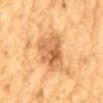workup=catalogued during a skin exam; not biopsied | patient=male, aged approximately 85 or older | location=the back | lesion diameter=≈6.5 mm | lighting=cross-polarized illumination | image=total-body-photography crop, ~15 mm field of view.